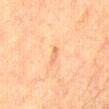Assessment: This lesion was catalogued during total-body skin photography and was not selected for biopsy. Background: A 15 mm close-up extracted from a 3D total-body photography capture. Captured under cross-polarized illumination. The lesion-visualizer software estimated a footprint of about 2 mm². It also reported an average lesion color of about L≈63 a*≈21 b*≈37 (CIELAB), roughly 7 lightness units darker than nearby skin, and a normalized lesion–skin contrast near 5. And it measured a border-irregularity index near 4.5/10, a within-lesion color-variation index near 0/10, and peripheral color asymmetry of about 0. It also reported an automated nevus-likeness rating near 0 out of 100 and a lesion-detection confidence of about 100/100. On the abdomen. A male subject, roughly 85 years of age or older. Longest diameter approximately 2.5 mm.